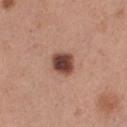workup=imaged on a skin check; not biopsied
anatomic site=the leg
TBP lesion metrics=an area of roughly 7 mm², an eccentricity of roughly 0.6, and two-axis asymmetry of about 0.2; a lesion color around L≈45 a*≈22 b*≈26 in CIELAB and a lesion–skin lightness drop of about 17; a border-irregularity index near 1.5/10 and a within-lesion color-variation index near 5/10
image source=~15 mm tile from a whole-body skin photo
subject=female, aged around 30
tile lighting=white-light
lesion size=~3 mm (longest diameter)